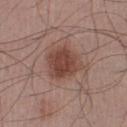Assessment:
Captured during whole-body skin photography for melanoma surveillance; the lesion was not biopsied.
Acquisition and patient details:
This is a white-light tile. The lesion's longest dimension is about 4.5 mm. The total-body-photography lesion software estimated an outline eccentricity of about 0.55 (0 = round, 1 = elongated) and two-axis asymmetry of about 0.25. The analysis additionally found a lesion color around L≈44 a*≈21 b*≈25 in CIELAB and a lesion-to-skin contrast of about 8.5 (normalized; higher = more distinct). And it measured border irregularity of about 2.5 on a 0–10 scale. The analysis additionally found a detector confidence of about 100 out of 100 that the crop contains a lesion. Cropped from a total-body skin-imaging series; the visible field is about 15 mm. The subject is a male aged 48 to 52. Located on the left lower leg.